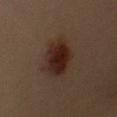Case summary:
– follow-up — catalogued during a skin exam; not biopsied
– automated lesion analysis — a lesion area of about 14 mm² and two-axis asymmetry of about 0.15
– image — ~15 mm crop, total-body skin-cancer survey
– site — the left upper arm
– lighting — cross-polarized illumination
– subject — female, in their 40s